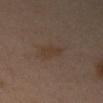Clinical impression: Recorded during total-body skin imaging; not selected for excision or biopsy. Background: A 15 mm close-up tile from a total-body photography series done for melanoma screening. This is a cross-polarized tile. About 2.5 mm across. The lesion is on the left arm. A female subject, aged approximately 40.